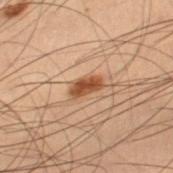Findings:
* follow-up · imaged on a skin check; not biopsied
* image · 15 mm crop, total-body photography
* illumination · cross-polarized illumination
* anatomic site · the right thigh
* subject · male, aged around 55